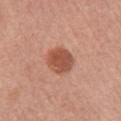Q: Was a biopsy performed?
A: catalogued during a skin exam; not biopsied
Q: How was this image acquired?
A: ~15 mm crop, total-body skin-cancer survey
Q: Who is the patient?
A: female, in their 60s
Q: Lesion location?
A: the right upper arm
Q: What lighting was used for the tile?
A: white-light